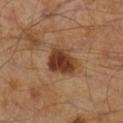Part of a total-body skin-imaging series; this lesion was reviewed on a skin check and was not flagged for biopsy. Measured at roughly 4 mm in maximum diameter. The lesion-visualizer software estimated an eccentricity of roughly 0.6 and a symmetry-axis asymmetry near 0.25. And it measured a lesion color around L≈37 a*≈21 b*≈31 in CIELAB and roughly 14 lightness units darker than nearby skin. It also reported a border-irregularity index near 2/10, internal color variation of about 5 on a 0–10 scale, and a peripheral color-asymmetry measure near 1.5. And it measured an automated nevus-likeness rating near 95 out of 100 and lesion-presence confidence of about 100/100. The tile uses cross-polarized illumination. A male subject, aged approximately 65. A region of skin cropped from a whole-body photographic capture, roughly 15 mm wide.